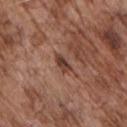{"lighting": "white-light", "patient": {"sex": "male", "age_approx": 70}, "image": {"source": "total-body photography crop", "field_of_view_mm": 15}, "site": "front of the torso", "lesion_size": {"long_diameter_mm_approx": 2.5}}